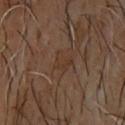Notes:
– biopsy status — total-body-photography surveillance lesion; no biopsy
– image source — ~15 mm crop, total-body skin-cancer survey
– TBP lesion metrics — a lesion area of about 4 mm²; a color-variation rating of about 1.5/10
– lighting — cross-polarized illumination
– anatomic site — the upper back
– lesion size — ≈2.5 mm
– subject — male, roughly 65 years of age